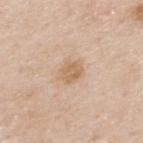Captured during whole-body skin photography for melanoma surveillance; the lesion was not biopsied.
A male subject aged around 80.
About 2.5 mm across.
The lesion is on the upper back.
Imaged with white-light lighting.
Cropped from a total-body skin-imaging series; the visible field is about 15 mm.